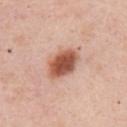| field | value |
|---|---|
| notes | catalogued during a skin exam; not biopsied |
| tile lighting | white-light illumination |
| site | the chest |
| subject | male, aged 48–52 |
| imaging modality | total-body-photography crop, ~15 mm field of view |
| lesion diameter | ~4.5 mm (longest diameter) |
| automated metrics | a mean CIELAB color near L≈55 a*≈25 b*≈31 and roughly 17 lightness units darker than nearby skin; border irregularity of about 1.5 on a 0–10 scale and a color-variation rating of about 5/10 |